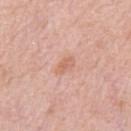follow-up: imaged on a skin check; not biopsied
patient: male, approximately 50 years of age
image source: total-body-photography crop, ~15 mm field of view
automated lesion analysis: two-axis asymmetry of about 0.15; a lesion-to-skin contrast of about 5.5 (normalized; higher = more distinct); a border-irregularity index near 1.5/10 and internal color variation of about 2.5 on a 0–10 scale
location: the front of the torso